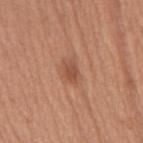Clinical impression:
Recorded during total-body skin imaging; not selected for excision or biopsy.
Acquisition and patient details:
A close-up tile cropped from a whole-body skin photograph, about 15 mm across. Located on the back. Measured at roughly 3 mm in maximum diameter. The subject is a male about 65 years old. This is a white-light tile. Automated image analysis of the tile measured a classifier nevus-likeness of about 70/100.